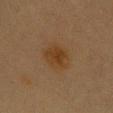• biopsy status: imaged on a skin check; not biopsied
• TBP lesion metrics: an area of roughly 8.5 mm² and an outline eccentricity of about 0.45 (0 = round, 1 = elongated); a mean CIELAB color near L≈33 a*≈16 b*≈30 and roughly 6 lightness units darker than nearby skin; internal color variation of about 3 on a 0–10 scale and radial color variation of about 1; a nevus-likeness score of about 90/100 and lesion-presence confidence of about 100/100
• illumination: cross-polarized illumination
• anatomic site: the front of the torso
• image source: ~15 mm crop, total-body skin-cancer survey
• subject: female, aged 38 to 42
• lesion size: ~3.5 mm (longest diameter)